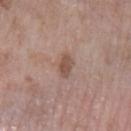Q: Was a biopsy performed?
A: total-body-photography surveillance lesion; no biopsy
Q: What is the imaging modality?
A: ~15 mm crop, total-body skin-cancer survey
Q: Where on the body is the lesion?
A: the left lower leg
Q: What are the patient's age and sex?
A: female, aged 73 to 77
Q: How was the tile lit?
A: white-light
Q: What is the lesion's diameter?
A: ~3 mm (longest diameter)
Q: What did automated image analysis measure?
A: an area of roughly 4 mm² and a shape eccentricity near 0.85; a lesion color around L≈51 a*≈18 b*≈25 in CIELAB and a normalized lesion–skin contrast near 7; a within-lesion color-variation index near 2/10 and radial color variation of about 0.5